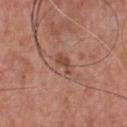The lesion was photographed on a routine skin check and not biopsied; there is no pathology result.
The total-body-photography lesion software estimated an area of roughly 3 mm² and a symmetry-axis asymmetry near 0.4.
A lesion tile, about 15 mm wide, cut from a 3D total-body photograph.
Captured under white-light illumination.
Located on the chest.
The patient is a male aged around 70.
Approximately 3 mm at its widest.